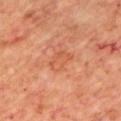Impression:
The lesion was photographed on a routine skin check and not biopsied; there is no pathology result.
Context:
About 3 mm across. This image is a 15 mm lesion crop taken from a total-body photograph. A male patient, about 70 years old. Located on the chest. Captured under cross-polarized illumination.